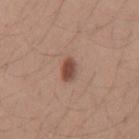Clinical impression: This lesion was catalogued during total-body skin photography and was not selected for biopsy. Acquisition and patient details: The subject is a male aged around 25. This is a white-light tile. A region of skin cropped from a whole-body photographic capture, roughly 15 mm wide. Measured at roughly 2.5 mm in maximum diameter. From the mid back. An algorithmic analysis of the crop reported a mean CIELAB color near L≈47 a*≈21 b*≈26, a lesion–skin lightness drop of about 13, and a normalized border contrast of about 9. And it measured a nevus-likeness score of about 95/100 and lesion-presence confidence of about 100/100.